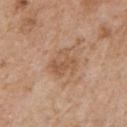workup: total-body-photography surveillance lesion; no biopsy | subject: male, aged 63 to 67 | size: ≈3 mm | imaging modality: 15 mm crop, total-body photography | tile lighting: white-light | automated lesion analysis: a footprint of about 6.5 mm², an outline eccentricity of about 0.55 (0 = round, 1 = elongated), and a symmetry-axis asymmetry near 0.25; roughly 7 lightness units darker than nearby skin and a normalized lesion–skin contrast near 5.5; a border-irregularity rating of about 3/10 and internal color variation of about 4 on a 0–10 scale; a lesion-detection confidence of about 100/100 | location: the chest.